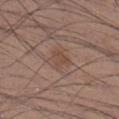Assessment: No biopsy was performed on this lesion — it was imaged during a full skin examination and was not determined to be concerning. Background: Cropped from a whole-body photographic skin survey; the tile spans about 15 mm. The lesion's longest dimension is about 3 mm. The subject is a male approximately 35 years of age. Located on the right lower leg. Imaged with white-light lighting.